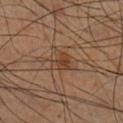Impression:
The lesion was photographed on a routine skin check and not biopsied; there is no pathology result.
Context:
The subject is a male in their 60s. A 15 mm crop from a total-body photograph taken for skin-cancer surveillance. The lesion is on the right lower leg.